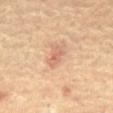biopsy_status: not biopsied; imaged during a skin examination
image:
  source: total-body photography crop
  field_of_view_mm: 15
lighting: cross-polarized
site: front of the torso
lesion_size:
  long_diameter_mm_approx: 3.0
patient:
  sex: male
  age_approx: 65